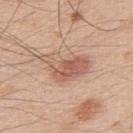The lesion was tiled from a total-body skin photograph and was not biopsied. The patient is a male aged approximately 50. Cropped from a whole-body photographic skin survey; the tile spans about 15 mm. Located on the upper back. This is a white-light tile.